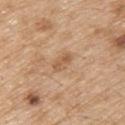biopsy_status: not biopsied; imaged during a skin examination
lighting: white-light
lesion_size:
  long_diameter_mm_approx: 3.0
image:
  source: total-body photography crop
  field_of_view_mm: 15
site: upper back
patient:
  sex: male
  age_approx: 70